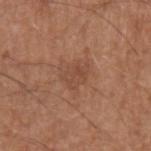Cropped from a whole-body photographic skin survey; the tile spans about 15 mm.
A male patient, approximately 65 years of age.
The lesion is located on the left upper arm.
Approximately 3.5 mm at its widest.